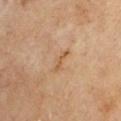This lesion was catalogued during total-body skin photography and was not selected for biopsy. A 15 mm close-up tile from a total-body photography series done for melanoma screening. On the arm. The total-body-photography lesion software estimated border irregularity of about 7.5 on a 0–10 scale, a within-lesion color-variation index near 0/10, and peripheral color asymmetry of about 0. The subject is a male aged 68 to 72. The tile uses cross-polarized illumination. Approximately 3 mm at its widest.